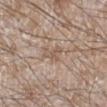Clinical summary: A male subject roughly 60 years of age. A lesion tile, about 15 mm wide, cut from a 3D total-body photograph. From the right lower leg.